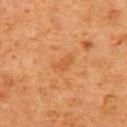Q: Was a biopsy performed?
A: catalogued during a skin exam; not biopsied
Q: What kind of image is this?
A: ~15 mm tile from a whole-body skin photo
Q: What is the anatomic site?
A: the upper back
Q: Automated lesion metrics?
A: border irregularity of about 3 on a 0–10 scale, a color-variation rating of about 1.5/10, and radial color variation of about 0.5
Q: What are the patient's age and sex?
A: female, aged 48–52